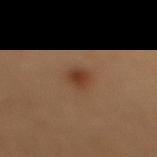{
  "biopsy_status": "not biopsied; imaged during a skin examination",
  "patient": {
    "sex": "female",
    "age_approx": 50
  },
  "image": {
    "source": "total-body photography crop",
    "field_of_view_mm": 15
  },
  "site": "upper back",
  "automated_metrics": {
    "area_mm2_approx": 4.0,
    "eccentricity": 0.8,
    "shape_asymmetry": 0.25,
    "border_irregularity_0_10": 2.5,
    "nevus_likeness_0_100": 100,
    "lesion_detection_confidence_0_100": 100
  },
  "lesion_size": {
    "long_diameter_mm_approx": 2.5
  }
}